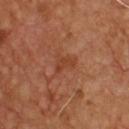• notes · imaged on a skin check; not biopsied
• illumination · cross-polarized illumination
• image · total-body-photography crop, ~15 mm field of view
• automated lesion analysis · a lesion area of about 2.5 mm², a shape eccentricity near 0.85, and two-axis asymmetry of about 0.45; an average lesion color of about L≈41 a*≈26 b*≈34 (CIELAB), about 7 CIELAB-L* units darker than the surrounding skin, and a normalized border contrast of about 6; border irregularity of about 4.5 on a 0–10 scale, a color-variation rating of about 0/10, and a peripheral color-asymmetry measure near 0
• size · ≈2.5 mm
• anatomic site · the chest
• patient · male, approximately 50 years of age